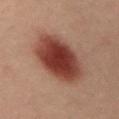<record>
<biopsy_status>not biopsied; imaged during a skin examination</biopsy_status>
<lesion_size>
  <long_diameter_mm_approx>7.0</long_diameter_mm_approx>
</lesion_size>
<lighting>cross-polarized</lighting>
<image>
  <source>total-body photography crop</source>
  <field_of_view_mm>15</field_of_view_mm>
</image>
<patient>
  <sex>male</sex>
  <age_approx>40</age_approx>
</patient>
<site>abdomen</site>
</record>